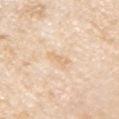Assessment: This lesion was catalogued during total-body skin photography and was not selected for biopsy. Background: A male patient, roughly 75 years of age. The recorded lesion diameter is about 3 mm. The total-body-photography lesion software estimated an area of roughly 3 mm², an outline eccentricity of about 0.95 (0 = round, 1 = elongated), and two-axis asymmetry of about 0.45. The software also gave an average lesion color of about L≈76 a*≈15 b*≈36 (CIELAB), a lesion–skin lightness drop of about 6, and a normalized border contrast of about 4.5. And it measured border irregularity of about 5 on a 0–10 scale and radial color variation of about 0. A region of skin cropped from a whole-body photographic capture, roughly 15 mm wide. Captured under white-light illumination. The lesion is on the right upper arm.